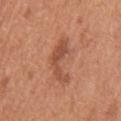follow-up: no biopsy performed (imaged during a skin exam) | image: ~15 mm tile from a whole-body skin photo | body site: the mid back | illumination: white-light illumination | diameter: about 5 mm | patient: male, approximately 65 years of age.